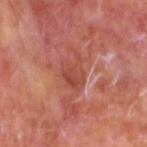<lesion>
<biopsy_status>not biopsied; imaged during a skin examination</biopsy_status>
<patient>
  <sex>male</sex>
  <age_approx>60</age_approx>
</patient>
<site>head or neck</site>
<image>
  <source>total-body photography crop</source>
  <field_of_view_mm>15</field_of_view_mm>
</image>
</lesion>